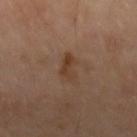biopsy status=imaged on a skin check; not biopsied
location=the left forearm
lesion size=≈3.5 mm
imaging modality=~15 mm tile from a whole-body skin photo
patient=female, approximately 55 years of age
image-analysis metrics=an average lesion color of about L≈39 a*≈18 b*≈29 (CIELAB), roughly 7 lightness units darker than nearby skin, and a normalized lesion–skin contrast near 7; a color-variation rating of about 3/10 and radial color variation of about 1; a classifier nevus-likeness of about 30/100 and a lesion-detection confidence of about 100/100